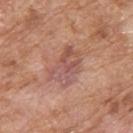biopsy status — total-body-photography surveillance lesion; no biopsy
acquisition — total-body-photography crop, ~15 mm field of view
image-analysis metrics — an outline eccentricity of about 0.75 (0 = round, 1 = elongated); a border-irregularity rating of about 3.5/10; a nevus-likeness score of about 0/100 and a detector confidence of about 100 out of 100 that the crop contains a lesion
lighting — white-light illumination
subject — male, aged 78 to 82
location — the upper back
diameter — ≈5 mm